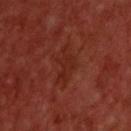follow-up=imaged on a skin check; not biopsied
lesion diameter=about 5 mm
patient=male, aged 58 to 62
anatomic site=the upper back
illumination=cross-polarized
imaging modality=~15 mm tile from a whole-body skin photo